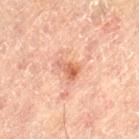notes=no biopsy performed (imaged during a skin exam); size=about 3 mm; imaging modality=total-body-photography crop, ~15 mm field of view; illumination=cross-polarized; location=the right leg; patient=female, aged 78 to 82.